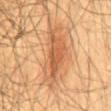Clinical impression: No biopsy was performed on this lesion — it was imaged during a full skin examination and was not determined to be concerning. Acquisition and patient details: From the abdomen. A lesion tile, about 15 mm wide, cut from a 3D total-body photograph. Captured under cross-polarized illumination. Measured at roughly 7.5 mm in maximum diameter. A male patient, aged 58–62. An algorithmic analysis of the crop reported an area of roughly 17 mm² and an outline eccentricity of about 0.9 (0 = round, 1 = elongated).